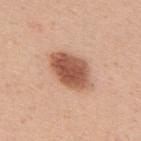<record>
  <biopsy_status>not biopsied; imaged during a skin examination</biopsy_status>
  <site>upper back</site>
  <image>
    <source>total-body photography crop</source>
    <field_of_view_mm>15</field_of_view_mm>
  </image>
  <lighting>white-light</lighting>
  <lesion_size>
    <long_diameter_mm_approx>5.5</long_diameter_mm_approx>
  </lesion_size>
  <patient>
    <sex>female</sex>
    <age_approx>30</age_approx>
  </patient>
</record>